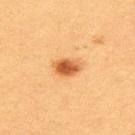The lesion was tiled from a total-body skin photograph and was not biopsied. Located on the upper back. Cropped from a whole-body photographic skin survey; the tile spans about 15 mm. A female patient in their 20s.